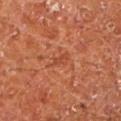workup: total-body-photography surveillance lesion; no biopsy | lesion size: ≈3 mm | body site: the left lower leg | automated metrics: a footprint of about 3.5 mm², an eccentricity of roughly 0.9, and a shape-asymmetry score of about 0.4 (0 = symmetric); an average lesion color of about L≈45 a*≈29 b*≈35 (CIELAB); an automated nevus-likeness rating near 0 out of 100 and lesion-presence confidence of about 95/100 | imaging modality: ~15 mm crop, total-body skin-cancer survey | subject: male, aged approximately 65.